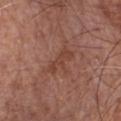Part of a total-body skin-imaging series; this lesion was reviewed on a skin check and was not flagged for biopsy.
Longest diameter approximately 3.5 mm.
A male subject, aged around 65.
The lesion is located on the chest.
Automated image analysis of the tile measured a lesion area of about 5 mm² and a symmetry-axis asymmetry near 0.35. And it measured a mean CIELAB color near L≈42 a*≈23 b*≈28, about 6 CIELAB-L* units darker than the surrounding skin, and a normalized border contrast of about 5.5. The analysis additionally found a border-irregularity rating of about 4.5/10, internal color variation of about 1 on a 0–10 scale, and a peripheral color-asymmetry measure near 0.
A 15 mm crop from a total-body photograph taken for skin-cancer surveillance.